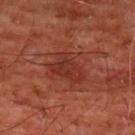Clinical impression: This lesion was catalogued during total-body skin photography and was not selected for biopsy.